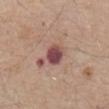Findings:
- follow-up — imaged on a skin check; not biopsied
- lesion diameter — ~3 mm (longest diameter)
- illumination — white-light illumination
- image source — 15 mm crop, total-body photography
- location — the abdomen
- image-analysis metrics — an area of roughly 5.5 mm² and two-axis asymmetry of about 0.1
- patient — male, aged approximately 80